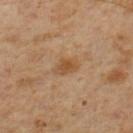Q: Was a biopsy performed?
A: total-body-photography surveillance lesion; no biopsy
Q: Who is the patient?
A: male, roughly 60 years of age
Q: What kind of image is this?
A: ~15 mm crop, total-body skin-cancer survey
Q: Lesion size?
A: ≈2.5 mm
Q: Lesion location?
A: the left lower leg